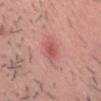Findings:
- notes · no biopsy performed (imaged during a skin exam)
- acquisition · ~15 mm crop, total-body skin-cancer survey
- site · the head or neck
- automated lesion analysis · an area of roughly 6.5 mm², an eccentricity of roughly 0.75, and two-axis asymmetry of about 0.2; an average lesion color of about L≈57 a*≈30 b*≈25 (CIELAB) and about 8 CIELAB-L* units darker than the surrounding skin; a within-lesion color-variation index near 2.5/10 and peripheral color asymmetry of about 0.5
- subject · male, roughly 30 years of age